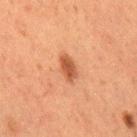This lesion was catalogued during total-body skin photography and was not selected for biopsy.
A female subject aged 48–52.
Imaged with cross-polarized lighting.
A region of skin cropped from a whole-body photographic capture, roughly 15 mm wide.
The recorded lesion diameter is about 3 mm.
On the right thigh.